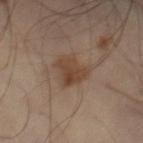workup: imaged on a skin check; not biopsied | tile lighting: cross-polarized illumination | acquisition: 15 mm crop, total-body photography | subject: male, approximately 50 years of age | site: the left leg.